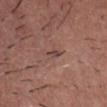notes=imaged on a skin check; not biopsied
tile lighting=white-light illumination
site=the chest
patient=male, roughly 60 years of age
imaging modality=total-body-photography crop, ~15 mm field of view